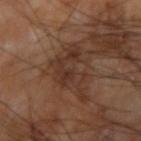The lesion was photographed on a routine skin check and not biopsied; there is no pathology result. A male patient about 65 years old. This image is a 15 mm lesion crop taken from a total-body photograph. The lesion-visualizer software estimated an area of roughly 12 mm², a shape eccentricity near 0.85, and a symmetry-axis asymmetry near 0.55. It also reported a lesion color around L≈33 a*≈18 b*≈26 in CIELAB and a normalized lesion–skin contrast near 6.5. And it measured border irregularity of about 9 on a 0–10 scale, a color-variation rating of about 4/10, and peripheral color asymmetry of about 1.5. Located on the right upper arm. Captured under cross-polarized illumination.